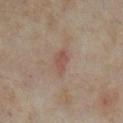workup: imaged on a skin check; not biopsied
patient: female, aged around 35
diameter: ≈3 mm
imaging modality: ~15 mm tile from a whole-body skin photo
site: the right lower leg
lighting: cross-polarized
image-analysis metrics: a lesion color around L≈48 a*≈18 b*≈23 in CIELAB, roughly 8 lightness units darker than nearby skin, and a lesion-to-skin contrast of about 6 (normalized; higher = more distinct); border irregularity of about 3 on a 0–10 scale, internal color variation of about 1 on a 0–10 scale, and radial color variation of about 0